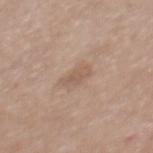follow-up=total-body-photography surveillance lesion; no biopsy | anatomic site=the mid back | subject=female, roughly 40 years of age | acquisition=15 mm crop, total-body photography.